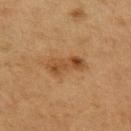Impression: This lesion was catalogued during total-body skin photography and was not selected for biopsy.